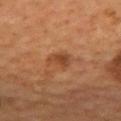<case>
<biopsy_status>not biopsied; imaged during a skin examination</biopsy_status>
<image>
  <source>total-body photography crop</source>
  <field_of_view_mm>15</field_of_view_mm>
</image>
<site>mid back</site>
<lesion_size>
  <long_diameter_mm_approx>2.5</long_diameter_mm_approx>
</lesion_size>
<patient>
  <sex>male</sex>
  <age_approx>55</age_approx>
</patient>
<automated_metrics>
  <cielab_L>41</cielab_L>
  <cielab_a>23</cielab_a>
  <cielab_b>33</cielab_b>
  <vs_skin_darker_L>8.0</vs_skin_darker_L>
  <vs_skin_contrast_norm>7.0</vs_skin_contrast_norm>
  <color_variation_0_10>3.0</color_variation_0_10>
  <peripheral_color_asymmetry>1.0</peripheral_color_asymmetry>
</automated_metrics>
</case>